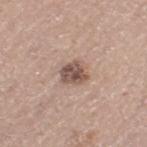Findings:
• image source — ~15 mm crop, total-body skin-cancer survey
• site — the left thigh
• illumination — white-light
• patient — male, in their mid-60s
• image-analysis metrics — an area of roughly 6 mm², a shape eccentricity near 0.55, and a shape-asymmetry score of about 0.25 (0 = symmetric)
• size — about 3 mm